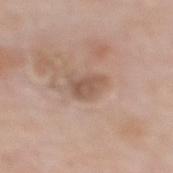No biopsy was performed on this lesion — it was imaged during a full skin examination and was not determined to be concerning. A female patient, aged 48 to 52. Cropped from a whole-body photographic skin survey; the tile spans about 15 mm. Located on the upper back.